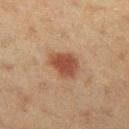This lesion was catalogued during total-body skin photography and was not selected for biopsy.
Automated image analysis of the tile measured an area of roughly 8.5 mm², an outline eccentricity of about 0.7 (0 = round, 1 = elongated), and two-axis asymmetry of about 0.25. And it measured a within-lesion color-variation index near 3.5/10 and peripheral color asymmetry of about 1. It also reported an automated nevus-likeness rating near 100 out of 100 and a detector confidence of about 100 out of 100 that the crop contains a lesion.
A female patient, aged 48–52.
A lesion tile, about 15 mm wide, cut from a 3D total-body photograph.
The lesion is on the left thigh.
The tile uses cross-polarized illumination.